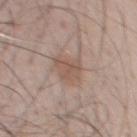notes — total-body-photography surveillance lesion; no biopsy
lighting — white-light
body site — the chest
lesion size — about 3.5 mm
subject — male, about 50 years old
automated metrics — a lesion color around L≈54 a*≈17 b*≈25 in CIELAB, roughly 8 lightness units darker than nearby skin, and a lesion-to-skin contrast of about 6 (normalized; higher = more distinct)
image — ~15 mm tile from a whole-body skin photo